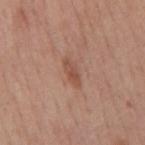Image and clinical context: Automated tile analysis of the lesion measured a lesion area of about 4 mm², an outline eccentricity of about 0.9 (0 = round, 1 = elongated), and a shape-asymmetry score of about 0.3 (0 = symmetric). Captured under white-light illumination. A male patient in their 60s. The lesion is located on the back. This image is a 15 mm lesion crop taken from a total-body photograph.